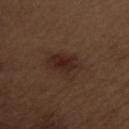Clinical impression: The lesion was tiled from a total-body skin photograph and was not biopsied. Image and clinical context: A male subject aged around 70. The lesion is located on the left upper arm. An algorithmic analysis of the crop reported border irregularity of about 3 on a 0–10 scale, a within-lesion color-variation index near 3.5/10, and peripheral color asymmetry of about 1. And it measured an automated nevus-likeness rating near 95 out of 100. This is a white-light tile. Approximately 4.5 mm at its widest. A lesion tile, about 15 mm wide, cut from a 3D total-body photograph.